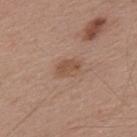<record>
<site>upper back</site>
<automated_metrics>
  <area_mm2_approx>5.0</area_mm2_approx>
  <eccentricity>0.7</eccentricity>
  <cielab_L>51</cielab_L>
  <cielab_a>19</cielab_a>
  <cielab_b>29</cielab_b>
  <vs_skin_darker_L>8.0</vs_skin_darker_L>
  <vs_skin_contrast_norm>6.5</vs_skin_contrast_norm>
  <border_irregularity_0_10>1.0</border_irregularity_0_10>
  <color_variation_0_10>2.5</color_variation_0_10>
  <peripheral_color_asymmetry>1.0</peripheral_color_asymmetry>
</automated_metrics>
<lesion_size>
  <long_diameter_mm_approx>2.5</long_diameter_mm_approx>
</lesion_size>
<patient>
  <sex>male</sex>
  <age_approx>55</age_approx>
</patient>
<image>
  <source>total-body photography crop</source>
  <field_of_view_mm>15</field_of_view_mm>
</image>
<lighting>white-light</lighting>
</record>